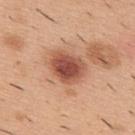Part of a total-body skin-imaging series; this lesion was reviewed on a skin check and was not flagged for biopsy.
A male patient, aged 38–42.
The lesion is on the upper back.
Cropped from a whole-body photographic skin survey; the tile spans about 15 mm.